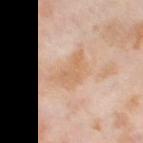Imaged during a routine full-body skin examination; the lesion was not biopsied and no histopathology is available.